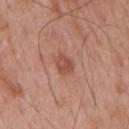Case summary:
• size — about 2.5 mm
• image — total-body-photography crop, ~15 mm field of view
• patient — male, aged around 55
• tile lighting — white-light
• image-analysis metrics — a lesion color around L≈50 a*≈25 b*≈29 in CIELAB and a normalized border contrast of about 6.5; a nevus-likeness score of about 65/100 and a lesion-detection confidence of about 100/100
• anatomic site — the mid back